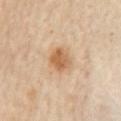workup: catalogued during a skin exam; not biopsied | image: 15 mm crop, total-body photography | body site: the chest | patient: female, aged around 60.